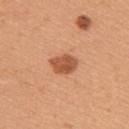Case summary:
– follow-up — catalogued during a skin exam; not biopsied
– diameter — about 3 mm
– patient — female, in their mid- to late 40s
– illumination — white-light
– image source — ~15 mm crop, total-body skin-cancer survey
– TBP lesion metrics — an area of roughly 7 mm², an eccentricity of roughly 0.65, and a symmetry-axis asymmetry near 0.2; an automated nevus-likeness rating near 95 out of 100 and a lesion-detection confidence of about 100/100
– location — the right upper arm